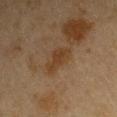biopsy status: total-body-photography surveillance lesion; no biopsy
patient: male, in their mid-60s
automated lesion analysis: a lesion area of about 5.5 mm², an outline eccentricity of about 0.85 (0 = round, 1 = elongated), and a shape-asymmetry score of about 0.4 (0 = symmetric); a border-irregularity rating of about 5/10 and radial color variation of about 0.5
anatomic site: the right upper arm
illumination: cross-polarized
image: 15 mm crop, total-body photography